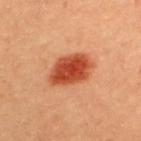Captured during whole-body skin photography for melanoma surveillance; the lesion was not biopsied. The lesion is on the upper back. About 5.5 mm across. A female patient, aged 33–37. Captured under cross-polarized illumination. A region of skin cropped from a whole-body photographic capture, roughly 15 mm wide.